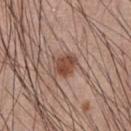Assessment: No biopsy was performed on this lesion — it was imaged during a full skin examination and was not determined to be concerning. Context: Approximately 3 mm at its widest. An algorithmic analysis of the crop reported an area of roughly 5.5 mm², an outline eccentricity of about 0.6 (0 = round, 1 = elongated), and a shape-asymmetry score of about 0.3 (0 = symmetric). The analysis additionally found a lesion color around L≈47 a*≈21 b*≈27 in CIELAB, roughly 12 lightness units darker than nearby skin, and a lesion-to-skin contrast of about 9 (normalized; higher = more distinct). The analysis additionally found a classifier nevus-likeness of about 90/100 and lesion-presence confidence of about 100/100. The lesion is located on the chest. A 15 mm crop from a total-body photograph taken for skin-cancer surveillance. A male patient, approximately 60 years of age.